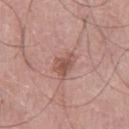Impression:
Captured during whole-body skin photography for melanoma surveillance; the lesion was not biopsied.
Image and clinical context:
A region of skin cropped from a whole-body photographic capture, roughly 15 mm wide. Imaged with white-light lighting. The lesion-visualizer software estimated a lesion area of about 5.5 mm² and a symmetry-axis asymmetry near 0.3. The software also gave internal color variation of about 3.5 on a 0–10 scale and radial color variation of about 1. The software also gave an automated nevus-likeness rating near 30 out of 100 and a lesion-detection confidence of about 100/100. A male patient, approximately 75 years of age. The lesion is located on the right thigh.